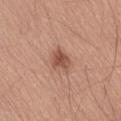Part of a total-body skin-imaging series; this lesion was reviewed on a skin check and was not flagged for biopsy. The lesion is located on the lower back. A male subject, aged around 55. Measured at roughly 3.5 mm in maximum diameter. A 15 mm close-up extracted from a 3D total-body photography capture. Captured under white-light illumination.